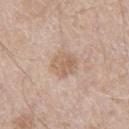Impression: This lesion was catalogued during total-body skin photography and was not selected for biopsy. Clinical summary: Located on the right thigh. This is a white-light tile. A male subject, in their mid- to late 60s. Cropped from a whole-body photographic skin survey; the tile spans about 15 mm.